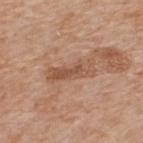{
  "biopsy_status": "not biopsied; imaged during a skin examination",
  "lesion_size": {
    "long_diameter_mm_approx": 5.5
  },
  "automated_metrics": {
    "border_irregularity_0_10": 4.0,
    "color_variation_0_10": 4.0,
    "peripheral_color_asymmetry": 1.5,
    "nevus_likeness_0_100": 0,
    "lesion_detection_confidence_0_100": 95
  },
  "image": {
    "source": "total-body photography crop",
    "field_of_view_mm": 15
  },
  "site": "upper back",
  "lighting": "white-light",
  "patient": {
    "sex": "male",
    "age_approx": 60
  }
}